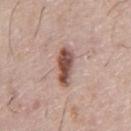notes=imaged on a skin check; not biopsied | patient=male, approximately 75 years of age | tile lighting=white-light illumination | size=≈4.5 mm | site=the abdomen | TBP lesion metrics=an area of roughly 7.5 mm², a shape eccentricity near 0.9, and two-axis asymmetry of about 0.2; a color-variation rating of about 4.5/10 and a peripheral color-asymmetry measure near 1.5; an automated nevus-likeness rating near 100 out of 100 and lesion-presence confidence of about 100/100 | acquisition=total-body-photography crop, ~15 mm field of view.